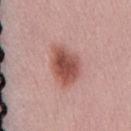<lesion>
<biopsy_status>not biopsied; imaged during a skin examination</biopsy_status>
</lesion>